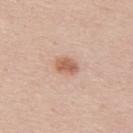Clinical impression:
Part of a total-body skin-imaging series; this lesion was reviewed on a skin check and was not flagged for biopsy.
Clinical summary:
On the mid back. A close-up tile cropped from a whole-body skin photograph, about 15 mm across. Captured under white-light illumination. An algorithmic analysis of the crop reported an average lesion color of about L≈60 a*≈22 b*≈31 (CIELAB), about 11 CIELAB-L* units darker than the surrounding skin, and a lesion-to-skin contrast of about 8 (normalized; higher = more distinct). The software also gave a border-irregularity index near 1.5/10 and a color-variation rating of about 3/10. The analysis additionally found a detector confidence of about 100 out of 100 that the crop contains a lesion. Longest diameter approximately 3 mm. The subject is a male in their mid- to late 40s.